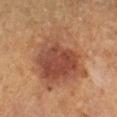notes: total-body-photography surveillance lesion; no biopsy
image source: total-body-photography crop, ~15 mm field of view
body site: the right lower leg
patient: female, aged approximately 60